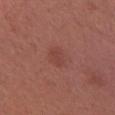Imaged during a routine full-body skin examination; the lesion was not biopsied and no histopathology is available. Approximately 2.5 mm at its widest. Located on the left forearm. This is a white-light tile. A lesion tile, about 15 mm wide, cut from a 3D total-body photograph. A female patient, roughly 35 years of age.